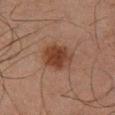The lesion was tiled from a total-body skin photograph and was not biopsied.
A male patient, about 65 years old.
Measured at roughly 4.5 mm in maximum diameter.
A 15 mm close-up tile from a total-body photography series done for melanoma screening.
This is a cross-polarized tile.
From the leg.
Automated image analysis of the tile measured a mean CIELAB color near L≈32 a*≈19 b*≈25 and about 10 CIELAB-L* units darker than the surrounding skin. It also reported a border-irregularity index near 2/10, a within-lesion color-variation index near 3/10, and a peripheral color-asymmetry measure near 1. And it measured a nevus-likeness score of about 95/100 and lesion-presence confidence of about 100/100.